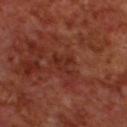| field | value |
|---|---|
| notes | total-body-photography surveillance lesion; no biopsy |
| patient | male, aged 68–72 |
| location | the upper back |
| image | total-body-photography crop, ~15 mm field of view |